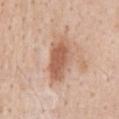  biopsy_status: not biopsied; imaged during a skin examination
  patient:
    sex: male
    age_approx: 60
  site: abdomen
  lighting: white-light
  lesion_size:
    long_diameter_mm_approx: 5.5
  image:
    source: total-body photography crop
    field_of_view_mm: 15